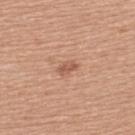Notes:
- biopsy status: total-body-photography surveillance lesion; no biopsy
- TBP lesion metrics: a lesion area of about 3 mm² and an eccentricity of roughly 0.85
- size: ≈2.5 mm
- image source: total-body-photography crop, ~15 mm field of view
- subject: female, in their mid-50s
- lighting: white-light illumination
- body site: the upper back